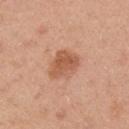{
  "biopsy_status": "not biopsied; imaged during a skin examination",
  "lighting": "white-light",
  "lesion_size": {
    "long_diameter_mm_approx": 4.0
  },
  "patient": {
    "sex": "male",
    "age_approx": 30
  },
  "site": "right upper arm",
  "image": {
    "source": "total-body photography crop",
    "field_of_view_mm": 15
  }
}